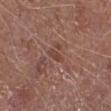Findings:
– notes: no biopsy performed (imaged during a skin exam)
– lighting: white-light
– image: ~15 mm tile from a whole-body skin photo
– patient: male, aged 73 to 77
– automated metrics: a lesion color around L≈43 a*≈21 b*≈25 in CIELAB, about 7 CIELAB-L* units darker than the surrounding skin, and a normalized border contrast of about 5.5; a border-irregularity rating of about 3/10 and a peripheral color-asymmetry measure near 0
– anatomic site: the right lower leg